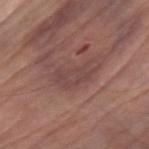Q: Is there a histopathology result?
A: total-body-photography surveillance lesion; no biopsy